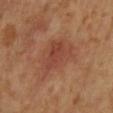Impression: Imaged during a routine full-body skin examination; the lesion was not biopsied and no histopathology is available. Image and clinical context: The patient is a male roughly 60 years of age. A region of skin cropped from a whole-body photographic capture, roughly 15 mm wide. The lesion is located on the mid back.